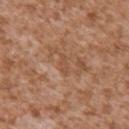Recorded during total-body skin imaging; not selected for excision or biopsy. The lesion's longest dimension is about 2.5 mm. A 15 mm close-up extracted from a 3D total-body photography capture. An algorithmic analysis of the crop reported a border-irregularity index near 3.5/10, internal color variation of about 0 on a 0–10 scale, and radial color variation of about 0. The software also gave a nevus-likeness score of about 0/100 and a lesion-detection confidence of about 95/100. The tile uses white-light illumination. The patient is a male in their mid- to late 40s. The lesion is located on the arm.